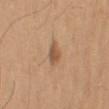Imaged during a routine full-body skin examination; the lesion was not biopsied and no histopathology is available.
The patient is a male aged 63 to 67.
Located on the right upper arm.
Cropped from a whole-body photographic skin survey; the tile spans about 15 mm.
Longest diameter approximately 2.5 mm.
This is a white-light tile.